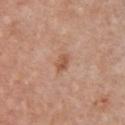{
  "automated_metrics": {
    "border_irregularity_0_10": 2.0,
    "color_variation_0_10": 2.5,
    "peripheral_color_asymmetry": 1.0
  },
  "site": "chest",
  "patient": {
    "sex": "female",
    "age_approx": 40
  },
  "image": {
    "source": "total-body photography crop",
    "field_of_view_mm": 15
  }
}